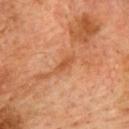lesion diameter = ≈3 mm
body site = the upper back
patient = male, aged 73–77
illumination = cross-polarized
image source = ~15 mm crop, total-body skin-cancer survey